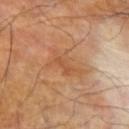  biopsy_status: not biopsied; imaged during a skin examination
  site: left forearm
  patient:
    sex: male
    age_approx: 70
  image:
    source: total-body photography crop
    field_of_view_mm: 15